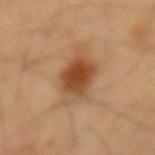The lesion was tiled from a total-body skin photograph and was not biopsied. The lesion is located on the back. A 15 mm crop from a total-body photograph taken for skin-cancer surveillance. A male patient, aged around 65. The lesion's longest dimension is about 6.5 mm.